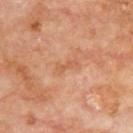Clinical summary: The tile uses cross-polarized illumination. The total-body-photography lesion software estimated a lesion color around L≈57 a*≈25 b*≈36 in CIELAB, a lesion–skin lightness drop of about 7, and a normalized lesion–skin contrast near 5. It also reported a border-irregularity index near 5/10, a within-lesion color-variation index near 0/10, and radial color variation of about 0. The analysis additionally found lesion-presence confidence of about 100/100. From the upper back. A male patient aged 68 to 72. Measured at roughly 3 mm in maximum diameter. A lesion tile, about 15 mm wide, cut from a 3D total-body photograph.